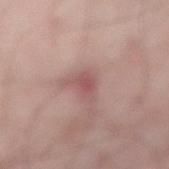Q: Is there a histopathology result?
A: catalogued during a skin exam; not biopsied
Q: What kind of image is this?
A: ~15 mm crop, total-body skin-cancer survey
Q: Patient demographics?
A: male, aged around 50
Q: Automated lesion metrics?
A: a mean CIELAB color near L≈51 a*≈23 b*≈20 and about 8 CIELAB-L* units darker than the surrounding skin; a lesion-detection confidence of about 95/100
Q: What is the lesion's diameter?
A: about 3.5 mm
Q: What is the anatomic site?
A: the abdomen
Q: How was the tile lit?
A: cross-polarized